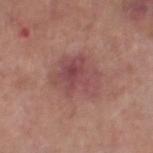The lesion was tiled from a total-body skin photograph and was not biopsied. A male patient, aged around 80. A close-up tile cropped from a whole-body skin photograph, about 15 mm across. The total-body-photography lesion software estimated a lesion color around L≈48 a*≈24 b*≈21 in CIELAB, about 8 CIELAB-L* units darker than the surrounding skin, and a normalized lesion–skin contrast near 6.5. It also reported a border-irregularity rating of about 2.5/10, a within-lesion color-variation index near 5.5/10, and radial color variation of about 1.5. Imaged with white-light lighting. From the right thigh. Longest diameter approximately 5.5 mm.